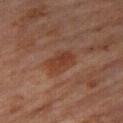Q: Was a biopsy performed?
A: no biopsy performed (imaged during a skin exam)
Q: Who is the patient?
A: male, aged approximately 60
Q: Where on the body is the lesion?
A: the right thigh
Q: What is the imaging modality?
A: total-body-photography crop, ~15 mm field of view